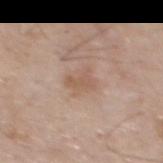Assessment:
The lesion was tiled from a total-body skin photograph and was not biopsied.
Acquisition and patient details:
An algorithmic analysis of the crop reported a footprint of about 4 mm² and an outline eccentricity of about 0.75 (0 = round, 1 = elongated). It also reported a mean CIELAB color near L≈56 a*≈18 b*≈28, a lesion–skin lightness drop of about 7, and a normalized lesion–skin contrast near 5.5. The tile uses white-light illumination. The patient is a male approximately 60 years of age. About 3 mm across. On the mid back. A close-up tile cropped from a whole-body skin photograph, about 15 mm across.